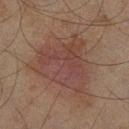Captured during whole-body skin photography for melanoma surveillance; the lesion was not biopsied.
Automated tile analysis of the lesion measured a lesion–skin lightness drop of about 6. And it measured a within-lesion color-variation index near 4/10 and radial color variation of about 1. The analysis additionally found a nevus-likeness score of about 0/100 and a detector confidence of about 100 out of 100 that the crop contains a lesion.
The tile uses cross-polarized illumination.
This image is a 15 mm lesion crop taken from a total-body photograph.
From the leg.
A male subject, aged 43 to 47.